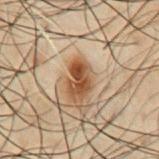Assessment: The lesion was photographed on a routine skin check and not biopsied; there is no pathology result. Context: A 15 mm close-up extracted from a 3D total-body photography capture. A male patient, in their 50s. The tile uses cross-polarized illumination. Located on the abdomen. The lesion's longest dimension is about 3.5 mm.